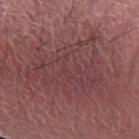Q: Is there a histopathology result?
A: total-body-photography surveillance lesion; no biopsy
Q: How was this image acquired?
A: ~15 mm crop, total-body skin-cancer survey
Q: Patient demographics?
A: male, about 75 years old
Q: What lighting was used for the tile?
A: white-light illumination
Q: What is the anatomic site?
A: the left forearm